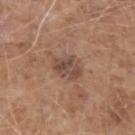Captured during whole-body skin photography for melanoma surveillance; the lesion was not biopsied. A close-up tile cropped from a whole-body skin photograph, about 15 mm across. The patient is a male roughly 60 years of age. The lesion is located on the arm. Longest diameter approximately 3 mm. Imaged with white-light lighting.